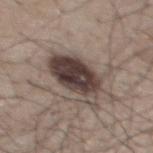acquisition = total-body-photography crop, ~15 mm field of view
patient = male, aged around 50
location = the back